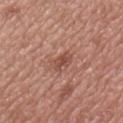Assessment: This lesion was catalogued during total-body skin photography and was not selected for biopsy. Clinical summary: The lesion is located on the mid back. About 2.5 mm across. The subject is a male about 65 years old. Cropped from a whole-body photographic skin survey; the tile spans about 15 mm. Automated tile analysis of the lesion measured an area of roughly 4 mm² and an outline eccentricity of about 0.75 (0 = round, 1 = elongated). The analysis additionally found a peripheral color-asymmetry measure near 1.5. The analysis additionally found an automated nevus-likeness rating near 0 out of 100 and a detector confidence of about 100 out of 100 that the crop contains a lesion.